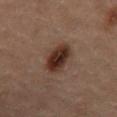Assessment:
No biopsy was performed on this lesion — it was imaged during a full skin examination and was not determined to be concerning.
Acquisition and patient details:
From the front of the torso. The patient is a male roughly 60 years of age. Automated tile analysis of the lesion measured a lesion area of about 9 mm², an eccentricity of roughly 0.65, and two-axis asymmetry of about 0.15. A close-up tile cropped from a whole-body skin photograph, about 15 mm across. Captured under cross-polarized illumination.